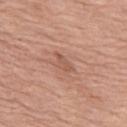Assessment: No biopsy was performed on this lesion — it was imaged during a full skin examination and was not determined to be concerning. Background: Located on the left thigh. Cropped from a whole-body photographic skin survey; the tile spans about 15 mm. Measured at roughly 3 mm in maximum diameter. The patient is a female roughly 65 years of age.